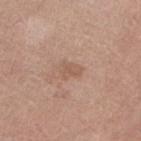biopsy status: catalogued during a skin exam; not biopsied | subject: female, in their mid-60s | image: total-body-photography crop, ~15 mm field of view | location: the left lower leg.